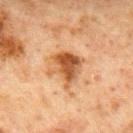Q: Was a biopsy performed?
A: no biopsy performed (imaged during a skin exam)
Q: Lesion location?
A: the mid back
Q: What did automated image analysis measure?
A: a mean CIELAB color near L≈48 a*≈22 b*≈36 and about 13 CIELAB-L* units darker than the surrounding skin; a border-irregularity rating of about 4/10, internal color variation of about 6.5 on a 0–10 scale, and peripheral color asymmetry of about 2; a lesion-detection confidence of about 100/100
Q: What is the imaging modality?
A: ~15 mm tile from a whole-body skin photo
Q: Who is the patient?
A: male, aged around 65
Q: What is the lesion's diameter?
A: ≈4.5 mm